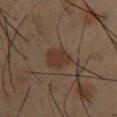| field | value |
|---|---|
| follow-up | imaged on a skin check; not biopsied |
| patient | male, aged around 40 |
| anatomic site | the upper back |
| image | ~15 mm tile from a whole-body skin photo |